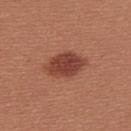  biopsy_status: not biopsied; imaged during a skin examination
  lesion_size:
    long_diameter_mm_approx: 5.0
  lighting: white-light
  automated_metrics:
    area_mm2_approx: 10.0
    eccentricity: 0.8
    shape_asymmetry: 0.2
    border_irregularity_0_10: 2.0
    color_variation_0_10: 2.5
    nevus_likeness_0_100: 95
    lesion_detection_confidence_0_100: 100
  image:
    source: total-body photography crop
    field_of_view_mm: 15
  patient:
    sex: female
    age_approx: 25
  site: upper back